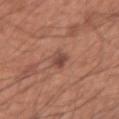follow-up = imaged on a skin check; not biopsied | location = the right upper arm | size = ~2.5 mm (longest diameter) | tile lighting = white-light illumination | imaging modality = total-body-photography crop, ~15 mm field of view | patient = male, roughly 60 years of age.